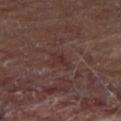Notes:
* workup — total-body-photography surveillance lesion; no biopsy
* illumination — cross-polarized illumination
* body site — the leg
* patient — male, aged 68 to 72
* imaging modality — ~15 mm crop, total-body skin-cancer survey